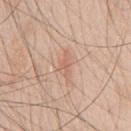Recorded during total-body skin imaging; not selected for excision or biopsy.